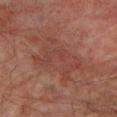A 15 mm close-up tile from a total-body photography series done for melanoma screening. A male patient, in their mid- to late 70s. The lesion's longest dimension is about 9 mm. This is a cross-polarized tile. The lesion is on the left lower leg.